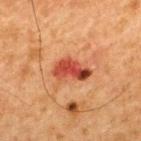Assessment: Imaged during a routine full-body skin examination; the lesion was not biopsied and no histopathology is available. Image and clinical context: This is a cross-polarized tile. On the upper back. A region of skin cropped from a whole-body photographic capture, roughly 15 mm wide. A male patient aged 63–67.